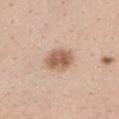The lesion was tiled from a total-body skin photograph and was not biopsied.